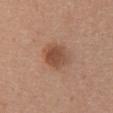Assessment: Captured during whole-body skin photography for melanoma surveillance; the lesion was not biopsied. Clinical summary: This image is a 15 mm lesion crop taken from a total-body photograph. Measured at roughly 4.5 mm in maximum diameter. Imaged with white-light lighting. A female patient, approximately 30 years of age. From the upper back.